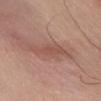notes=no biopsy performed (imaged during a skin exam)
lighting=white-light
acquisition=total-body-photography crop, ~15 mm field of view
subject=male, in their mid- to late 50s
diameter=about 5.5 mm
anatomic site=the right upper arm